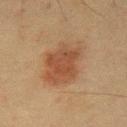lighting: cross-polarized
patient: male, aged 58 to 62
size: ~6 mm (longest diameter)
TBP lesion metrics: a within-lesion color-variation index near 3.5/10
image: ~15 mm tile from a whole-body skin photo
location: the front of the torso